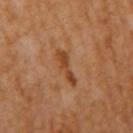Part of a total-body skin-imaging series; this lesion was reviewed on a skin check and was not flagged for biopsy.